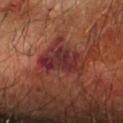This lesion was catalogued during total-body skin photography and was not selected for biopsy. A 15 mm close-up extracted from a 3D total-body photography capture. The lesion is on the left forearm. A male subject, aged around 60. Longest diameter approximately 5 mm.